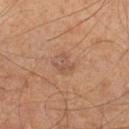The lesion was tiled from a total-body skin photograph and was not biopsied. The lesion is located on the left lower leg. A subject in their mid- to late 50s. The tile uses cross-polarized illumination. Measured at roughly 2.5 mm in maximum diameter. Cropped from a total-body skin-imaging series; the visible field is about 15 mm.